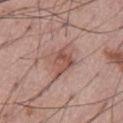follow-up: no biopsy performed (imaged during a skin exam) | site: the abdomen | image: 15 mm crop, total-body photography | subject: male, roughly 55 years of age.